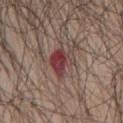Q: Was a biopsy performed?
A: no biopsy performed (imaged during a skin exam)
Q: Automated lesion metrics?
A: a lesion area of about 7.5 mm² and a shape eccentricity near 0.65; a nevus-likeness score of about 5/100
Q: Who is the patient?
A: male, about 70 years old
Q: What is the imaging modality?
A: total-body-photography crop, ~15 mm field of view
Q: What is the lesion's diameter?
A: ~3.5 mm (longest diameter)
Q: How was the tile lit?
A: white-light
Q: Where on the body is the lesion?
A: the mid back